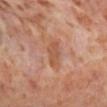Impression: Recorded during total-body skin imaging; not selected for excision or biopsy.